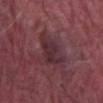Clinical impression:
The lesion was tiled from a total-body skin photograph and was not biopsied.
Context:
The recorded lesion diameter is about 5.5 mm. Located on the abdomen. A 15 mm close-up tile from a total-body photography series done for melanoma screening. This is a white-light tile. A male subject aged 38 to 42.